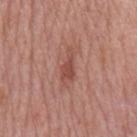The lesion was photographed on a routine skin check and not biopsied; there is no pathology result. Imaged with white-light lighting. About 4 mm across. A male subject about 65 years old. The lesion is on the mid back. This image is a 15 mm lesion crop taken from a total-body photograph.